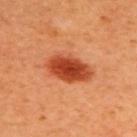Q: Is there a histopathology result?
A: total-body-photography surveillance lesion; no biopsy
Q: What lighting was used for the tile?
A: cross-polarized
Q: What is the lesion's diameter?
A: about 5.5 mm
Q: What is the imaging modality?
A: ~15 mm crop, total-body skin-cancer survey
Q: What is the anatomic site?
A: the upper back
Q: Who is the patient?
A: female, aged 43 to 47